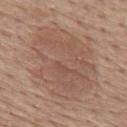Clinical impression: The lesion was photographed on a routine skin check and not biopsied; there is no pathology result. Background: A female patient, aged 63–67. The lesion is on the upper back. Imaged with white-light lighting. Approximately 10 mm at its widest. Cropped from a total-body skin-imaging series; the visible field is about 15 mm.